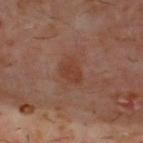The lesion was photographed on a routine skin check and not biopsied; there is no pathology result.
The total-body-photography lesion software estimated a border-irregularity index near 2.5/10, internal color variation of about 1 on a 0–10 scale, and a peripheral color-asymmetry measure near 0.5. It also reported a classifier nevus-likeness of about 0/100.
The subject is a male aged 58 to 62.
The lesion's longest dimension is about 3 mm.
On the upper back.
Cropped from a total-body skin-imaging series; the visible field is about 15 mm.